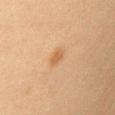Case summary:
– biopsy status — total-body-photography surveillance lesion; no biopsy
– tile lighting — cross-polarized illumination
– subject — female, aged approximately 40
– anatomic site — the left upper arm
– TBP lesion metrics — a lesion area of about 3 mm² and a shape-asymmetry score of about 0.25 (0 = symmetric); a lesion color around L≈50 a*≈18 b*≈34 in CIELAB; a border-irregularity rating of about 2.5/10, a within-lesion color-variation index near 1/10, and peripheral color asymmetry of about 0.5; an automated nevus-likeness rating near 80 out of 100 and lesion-presence confidence of about 100/100
– image source — 15 mm crop, total-body photography
– lesion diameter — about 2.5 mm